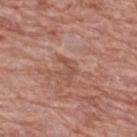No biopsy was performed on this lesion — it was imaged during a full skin examination and was not determined to be concerning. The recorded lesion diameter is about 3 mm. The subject is a female about 60 years old. Cropped from a total-body skin-imaging series; the visible field is about 15 mm. On the upper back. An algorithmic analysis of the crop reported a lesion-detection confidence of about 90/100. Imaged with white-light lighting.